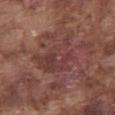Acquisition and patient details:
A 15 mm close-up tile from a total-body photography series done for melanoma screening. Imaged with white-light lighting. The lesion is located on the front of the torso. A male patient, about 75 years old. An algorithmic analysis of the crop reported a mean CIELAB color near L≈39 a*≈23 b*≈21, a lesion–skin lightness drop of about 7, and a lesion-to-skin contrast of about 6.5 (normalized; higher = more distinct). Approximately 6 mm at its widest.